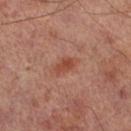| feature | finding |
|---|---|
| workup | imaged on a skin check; not biopsied |
| anatomic site | the right lower leg |
| patient | male, aged 63–67 |
| imaging modality | ~15 mm crop, total-body skin-cancer survey |